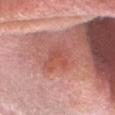Assessment: Recorded during total-body skin imaging; not selected for excision or biopsy. Image and clinical context: The patient is a male aged 53 to 57. From the head or neck. Imaged with white-light lighting. A region of skin cropped from a whole-body photographic capture, roughly 15 mm wide. The lesion's longest dimension is about 4 mm. Automated tile analysis of the lesion measured a border-irregularity rating of about 3.5/10, a within-lesion color-variation index near 4/10, and a peripheral color-asymmetry measure near 1.5.